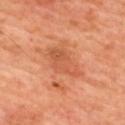<lesion>
<biopsy_status>not biopsied; imaged during a skin examination</biopsy_status>
<site>upper back</site>
<image>
  <source>total-body photography crop</source>
  <field_of_view_mm>15</field_of_view_mm>
</image>
<patient>
  <sex>male</sex>
  <age_approx>60</age_approx>
</patient>
<lighting>cross-polarized</lighting>
</lesion>